The subject is a male aged 38–42; a 15 mm close-up extracted from a 3D total-body photography capture; the lesion is located on the upper back:
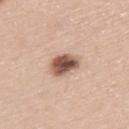Q: What is the lesion's diameter?
A: ≈3.5 mm
Q: What did pathology find?
A: a dysplastic (Clark) nevus